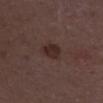Captured during whole-body skin photography for melanoma surveillance; the lesion was not biopsied. This is a white-light tile. Located on the left lower leg. A female patient, in their mid- to late 30s. A region of skin cropped from a whole-body photographic capture, roughly 15 mm wide. Automated image analysis of the tile measured a lesion color around L≈27 a*≈16 b*≈19 in CIELAB, about 8 CIELAB-L* units darker than the surrounding skin, and a lesion-to-skin contrast of about 8.5 (normalized; higher = more distinct). And it measured a border-irregularity rating of about 2/10 and internal color variation of about 2.5 on a 0–10 scale. And it measured a nevus-likeness score of about 60/100 and a lesion-detection confidence of about 100/100.